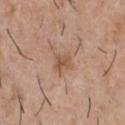No biopsy was performed on this lesion — it was imaged during a full skin examination and was not determined to be concerning. The patient is a male aged around 30. Approximately 2.5 mm at its widest. Located on the chest. Imaged with white-light lighting. A lesion tile, about 15 mm wide, cut from a 3D total-body photograph.